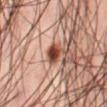Imaged during a routine full-body skin examination; the lesion was not biopsied and no histopathology is available. A male subject aged around 45. About 2.5 mm across. From the front of the torso. A 15 mm crop from a total-body photograph taken for skin-cancer surveillance. Automated tile analysis of the lesion measured a lesion–skin lightness drop of about 18 and a lesion-to-skin contrast of about 13.5 (normalized; higher = more distinct). The analysis additionally found a lesion-detection confidence of about 100/100. The tile uses cross-polarized illumination.